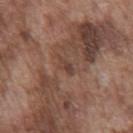Captured during whole-body skin photography for melanoma surveillance; the lesion was not biopsied. Located on the chest. The lesion-visualizer software estimated an average lesion color of about L≈41 a*≈19 b*≈24 (CIELAB), a lesion–skin lightness drop of about 7, and a normalized border contrast of about 6. About 2.5 mm across. The tile uses white-light illumination. A 15 mm close-up tile from a total-body photography series done for melanoma screening. A male patient, about 75 years old.